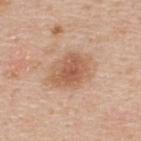No biopsy was performed on this lesion — it was imaged during a full skin examination and was not determined to be concerning.
A male patient aged 33–37.
A region of skin cropped from a whole-body photographic capture, roughly 15 mm wide.
Approximately 5 mm at its widest.
The total-body-photography lesion software estimated a lesion area of about 13 mm² and an eccentricity of roughly 0.7. The software also gave a classifier nevus-likeness of about 55/100 and a lesion-detection confidence of about 100/100.
The lesion is on the upper back.
The tile uses white-light illumination.